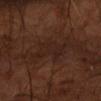<case>
  <biopsy_status>not biopsied; imaged during a skin examination</biopsy_status>
  <site>left forearm</site>
  <lesion_size>
    <long_diameter_mm_approx>5.0</long_diameter_mm_approx>
  </lesion_size>
  <patient>
    <sex>male</sex>
    <age_approx>55</age_approx>
  </patient>
  <image>
    <source>total-body photography crop</source>
    <field_of_view_mm>15</field_of_view_mm>
  </image>
  <lighting>cross-polarized</lighting>
</case>